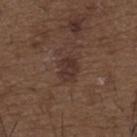| field | value |
|---|---|
| notes | imaged on a skin check; not biopsied |
| subject | male, aged 48 to 52 |
| image-analysis metrics | a lesion color around L≈32 a*≈17 b*≈21 in CIELAB, a lesion–skin lightness drop of about 7, and a normalized border contrast of about 7; a border-irregularity index near 2/10, internal color variation of about 2.5 on a 0–10 scale, and a peripheral color-asymmetry measure near 1 |
| acquisition | total-body-photography crop, ~15 mm field of view |
| illumination | white-light |
| body site | the upper back |